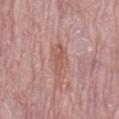Notes:
- workup — no biopsy performed (imaged during a skin exam)
- imaging modality — ~15 mm crop, total-body skin-cancer survey
- illumination — white-light
- diameter — ≈4 mm
- anatomic site — the left thigh
- automated metrics — an area of roughly 6.5 mm², an eccentricity of roughly 0.9, and a shape-asymmetry score of about 0.3 (0 = symmetric); a lesion-to-skin contrast of about 5.5 (normalized; higher = more distinct); a border-irregularity rating of about 3.5/10 and a peripheral color-asymmetry measure near 1
- patient — female, aged 63–67